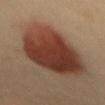Part of a total-body skin-imaging series; this lesion was reviewed on a skin check and was not flagged for biopsy. A 15 mm close-up tile from a total-body photography series done for melanoma screening. On the back. The patient is a male roughly 40 years of age.